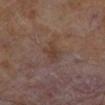{"biopsy_status": "not biopsied; imaged during a skin examination", "patient": {"sex": "male", "age_approx": 65}, "image": {"source": "total-body photography crop", "field_of_view_mm": 15}, "lesion_size": {"long_diameter_mm_approx": 3.0}, "automated_metrics": {"border_irregularity_0_10": 3.0, "color_variation_0_10": 1.0, "peripheral_color_asymmetry": 0.0}, "lighting": "cross-polarized"}